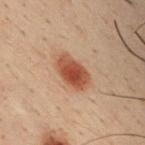Q: Was a biopsy performed?
A: imaged on a skin check; not biopsied
Q: Lesion size?
A: ≈4 mm
Q: What did automated image analysis measure?
A: a lesion area of about 9.5 mm², an eccentricity of roughly 0.8, and a shape-asymmetry score of about 0.15 (0 = symmetric); an automated nevus-likeness rating near 100 out of 100 and lesion-presence confidence of about 100/100
Q: What kind of image is this?
A: total-body-photography crop, ~15 mm field of view
Q: Patient demographics?
A: male, about 30 years old
Q: Where on the body is the lesion?
A: the chest
Q: How was the tile lit?
A: cross-polarized illumination